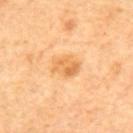Q: Was this lesion biopsied?
A: catalogued during a skin exam; not biopsied
Q: What lighting was used for the tile?
A: cross-polarized
Q: What is the anatomic site?
A: the back
Q: Who is the patient?
A: female, in their mid-60s
Q: What kind of image is this?
A: ~15 mm tile from a whole-body skin photo